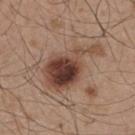follow-up — catalogued during a skin exam; not biopsied | patient — male, aged 48–52 | anatomic site — the upper back | image — 15 mm crop, total-body photography.